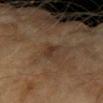Clinical impression:
No biopsy was performed on this lesion — it was imaged during a full skin examination and was not determined to be concerning.
Acquisition and patient details:
The subject is a female in their 60s. The lesion is located on the right forearm. A close-up tile cropped from a whole-body skin photograph, about 15 mm across.